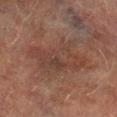Captured during whole-body skin photography for melanoma surveillance; the lesion was not biopsied. Located on the leg. The lesion's longest dimension is about 7 mm. Imaged with cross-polarized lighting. A male patient, aged around 75. Cropped from a total-body skin-imaging series; the visible field is about 15 mm.